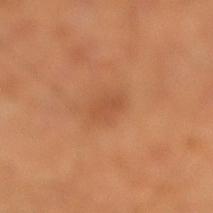The lesion is on the right lower leg. Cropped from a total-body skin-imaging series; the visible field is about 15 mm. A male subject aged around 55. The lesion's longest dimension is about 3 mm. Automated tile analysis of the lesion measured a mean CIELAB color near L≈50 a*≈25 b*≈36 and a normalized lesion–skin contrast near 4.5. And it measured internal color variation of about 2 on a 0–10 scale and radial color variation of about 1. The tile uses cross-polarized illumination.